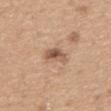Impression:
The lesion was tiled from a total-body skin photograph and was not biopsied.
Context:
A male subject approximately 65 years of age. Imaged with white-light lighting. Measured at roughly 2.5 mm in maximum diameter. A roughly 15 mm field-of-view crop from a total-body skin photograph. The lesion is located on the upper back.